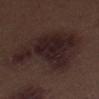• workup: no biopsy performed (imaged during a skin exam)
• patient: male, aged 68–72
• image source: ~15 mm crop, total-body skin-cancer survey
• illumination: white-light illumination
• body site: the right thigh
• automated metrics: a lesion area of about 39 mm², an outline eccentricity of about 0.8 (0 = round, 1 = elongated), and a symmetry-axis asymmetry near 0.45; an average lesion color of about L≈21 a*≈14 b*≈14 (CIELAB), a lesion–skin lightness drop of about 8, and a normalized border contrast of about 10.5
• diameter: ≈9 mm